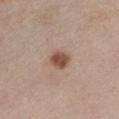Part of a total-body skin-imaging series; this lesion was reviewed on a skin check and was not flagged for biopsy. A female subject, in their mid-60s. Measured at roughly 3 mm in maximum diameter. The tile uses white-light illumination. Located on the chest. Automated tile analysis of the lesion measured an average lesion color of about L≈51 a*≈20 b*≈28 (CIELAB), a lesion–skin lightness drop of about 13, and a lesion-to-skin contrast of about 9.5 (normalized; higher = more distinct). And it measured a border-irregularity rating of about 1.5/10 and radial color variation of about 1. It also reported a nevus-likeness score of about 100/100 and lesion-presence confidence of about 100/100. A lesion tile, about 15 mm wide, cut from a 3D total-body photograph.